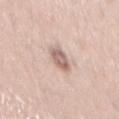Q: Is there a histopathology result?
A: imaged on a skin check; not biopsied
Q: What lighting was used for the tile?
A: white-light illumination
Q: Where on the body is the lesion?
A: the mid back
Q: What is the lesion's diameter?
A: ~4 mm (longest diameter)
Q: What are the patient's age and sex?
A: male, aged around 40
Q: How was this image acquired?
A: total-body-photography crop, ~15 mm field of view
Q: What did automated image analysis measure?
A: a footprint of about 6 mm², an outline eccentricity of about 0.9 (0 = round, 1 = elongated), and a symmetry-axis asymmetry near 0.15; an average lesion color of about L≈64 a*≈17 b*≈24 (CIELAB), about 13 CIELAB-L* units darker than the surrounding skin, and a normalized border contrast of about 7.5; a lesion-detection confidence of about 95/100